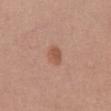Background: Located on the front of the torso. An algorithmic analysis of the crop reported an area of roughly 4 mm², a shape eccentricity near 0.65, and a symmetry-axis asymmetry near 0.15. The software also gave an average lesion color of about L≈54 a*≈23 b*≈30 (CIELAB), a lesion–skin lightness drop of about 9, and a normalized lesion–skin contrast near 7. The analysis additionally found a nevus-likeness score of about 90/100 and lesion-presence confidence of about 100/100. Imaged with white-light lighting. The lesion's longest dimension is about 2.5 mm. A female patient, aged 58–62. A roughly 15 mm field-of-view crop from a total-body skin photograph.